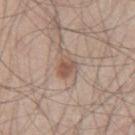Findings:
* notes — catalogued during a skin exam; not biopsied
* site — the right thigh
* patient — male, aged 43–47
* imaging modality — ~15 mm tile from a whole-body skin photo
* illumination — white-light illumination